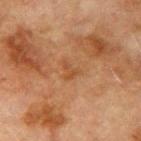  biopsy_status: not biopsied; imaged during a skin examination
  lesion_size:
    long_diameter_mm_approx: 2.5
  site: right upper arm
  image:
    source: total-body photography crop
    field_of_view_mm: 15
  automated_metrics:
    area_mm2_approx: 3.0
    cielab_L: 41
    cielab_a: 20
    cielab_b: 32
    vs_skin_darker_L: 5.0
    vs_skin_contrast_norm: 5.0
  lighting: cross-polarized
  patient:
    sex: male
    age_approx: 75